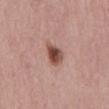<case>
  <patient>
    <sex>female</sex>
    <age_approx>65</age_approx>
  </patient>
  <image>
    <source>total-body photography crop</source>
    <field_of_view_mm>15</field_of_view_mm>
  </image>
  <site>abdomen</site>
  <lighting>white-light</lighting>
  <lesion_size>
    <long_diameter_mm_approx>3.0</long_diameter_mm_approx>
  </lesion_size>
</case>